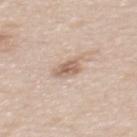Part of a total-body skin-imaging series; this lesion was reviewed on a skin check and was not flagged for biopsy. The subject is a female aged around 45. The lesion-visualizer software estimated a lesion area of about 4.5 mm², a shape eccentricity near 0.6, and a symmetry-axis asymmetry near 0.25. The analysis additionally found an automated nevus-likeness rating near 35 out of 100. A 15 mm close-up extracted from a 3D total-body photography capture. The lesion is located on the back.